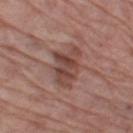<record>
  <biopsy_status>not biopsied; imaged during a skin examination</biopsy_status>
  <automated_metrics>
    <area_mm2_approx>11.0</area_mm2_approx>
    <eccentricity>0.75</eccentricity>
    <shape_asymmetry>0.35</shape_asymmetry>
  </automated_metrics>
  <site>left thigh</site>
  <patient>
    <sex>male</sex>
    <age_approx>70</age_approx>
  </patient>
  <lesion_size>
    <long_diameter_mm_approx>4.5</long_diameter_mm_approx>
  </lesion_size>
  <image>
    <source>total-body photography crop</source>
    <field_of_view_mm>15</field_of_view_mm>
  </image>
</record>